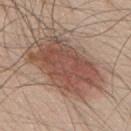notes: catalogued during a skin exam; not biopsied | anatomic site: the upper back | imaging modality: ~15 mm tile from a whole-body skin photo | patient: male, in their 50s | lighting: white-light illumination.